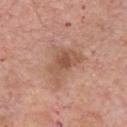This lesion was catalogued during total-body skin photography and was not selected for biopsy. A male patient aged approximately 75. From the front of the torso. A 15 mm close-up extracted from a 3D total-body photography capture. The recorded lesion diameter is about 5 mm. Automated image analysis of the tile measured a footprint of about 11 mm², an eccentricity of roughly 0.8, and a shape-asymmetry score of about 0.4 (0 = symmetric). It also reported a lesion color around L≈54 a*≈21 b*≈30 in CIELAB and a lesion-to-skin contrast of about 6.5 (normalized; higher = more distinct). The software also gave a nevus-likeness score of about 20/100 and a lesion-detection confidence of about 100/100.